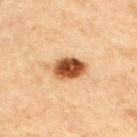Clinical impression: The lesion was photographed on a routine skin check and not biopsied; there is no pathology result.